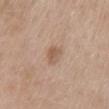Impression:
No biopsy was performed on this lesion — it was imaged during a full skin examination and was not determined to be concerning.
Image and clinical context:
This image is a 15 mm lesion crop taken from a total-body photograph. Captured under white-light illumination. The patient is a female in their 60s. From the mid back.